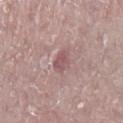Assessment: No biopsy was performed on this lesion — it was imaged during a full skin examination and was not determined to be concerning. Background: A female subject aged 68 to 72. A region of skin cropped from a whole-body photographic capture, roughly 15 mm wide. Located on the right lower leg.